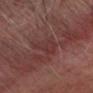Captured during whole-body skin photography for melanoma surveillance; the lesion was not biopsied. The tile uses cross-polarized illumination. A male patient, roughly 60 years of age. Automated image analysis of the tile measured an average lesion color of about L≈33 a*≈21 b*≈19 (CIELAB) and a normalized border contrast of about 5. The analysis additionally found a nevus-likeness score of about 0/100. A 15 mm close-up tile from a total-body photography series done for melanoma screening. The lesion is on the head or neck. Longest diameter approximately 3.5 mm.